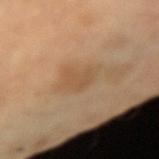biopsy status = imaged on a skin check; not biopsied | illumination = cross-polarized | image = ~15 mm crop, total-body skin-cancer survey | site = the left arm | subject = female, aged 58–62 | lesion diameter = ≈2.5 mm.